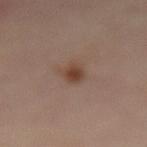Impression:
No biopsy was performed on this lesion — it was imaged during a full skin examination and was not determined to be concerning.
Clinical summary:
A female subject, about 50 years old. The lesion is on the abdomen. Automated tile analysis of the lesion measured a lesion–skin lightness drop of about 8 and a lesion-to-skin contrast of about 7.5 (normalized; higher = more distinct). It also reported border irregularity of about 2.5 on a 0–10 scale and a within-lesion color-variation index near 4/10. And it measured a nevus-likeness score of about 90/100 and a detector confidence of about 100 out of 100 that the crop contains a lesion. A 15 mm close-up extracted from a 3D total-body photography capture. Imaged with cross-polarized lighting.